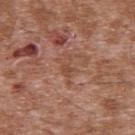notes = catalogued during a skin exam; not biopsied
body site = the upper back
image source = 15 mm crop, total-body photography
tile lighting = white-light illumination
patient = male, aged 43–47